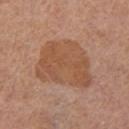lesion_size:
  long_diameter_mm_approx: 7.0
site: left thigh
patient:
  sex: female
  age_approx: 55
automated_metrics:
  area_mm2_approx: 29.0
  eccentricity: 0.6
  cielab_L: 52
  cielab_a: 21
  cielab_b: 32
  vs_skin_darker_L: 8.0
  border_irregularity_0_10: 3.0
  color_variation_0_10: 3.0
  peripheral_color_asymmetry: 1.0
  nevus_likeness_0_100: 5
  lesion_detection_confidence_0_100: 100
image:
  source: total-body photography crop
  field_of_view_mm: 15